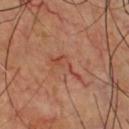Case summary:
• anatomic site: the chest
• lesion diameter: about 4.5 mm
• image source: ~15 mm tile from a whole-body skin photo
• subject: male, aged around 60
• automated lesion analysis: a lesion color around L≈45 a*≈27 b*≈30 in CIELAB, a lesion–skin lightness drop of about 7, and a normalized border contrast of about 5.5
• tile lighting: cross-polarized illumination